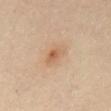Impression:
No biopsy was performed on this lesion — it was imaged during a full skin examination and was not determined to be concerning.
Acquisition and patient details:
A female subject, approximately 40 years of age. The lesion's longest dimension is about 3.5 mm. The lesion is on the abdomen. A 15 mm crop from a total-body photograph taken for skin-cancer surveillance.